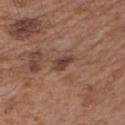Findings:
• biopsy status · imaged on a skin check; not biopsied
• location · the left upper arm
• lesion diameter · about 3 mm
• tile lighting · white-light illumination
• patient · male, approximately 55 years of age
• imaging modality · 15 mm crop, total-body photography